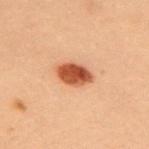A female patient, aged 28 to 32.
The lesion is located on the upper back.
Approximately 4.5 mm at its widest.
Cropped from a whole-body photographic skin survey; the tile spans about 15 mm.
Imaged with cross-polarized lighting.